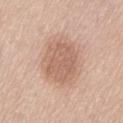{"biopsy_status": "not biopsied; imaged during a skin examination", "image": {"source": "total-body photography crop", "field_of_view_mm": 15}, "patient": {"sex": "female", "age_approx": 65}, "automated_metrics": {"area_mm2_approx": 17.0, "eccentricity": 0.65, "shape_asymmetry": 0.15, "color_variation_0_10": 2.5, "peripheral_color_asymmetry": 1.0, "nevus_likeness_0_100": 20, "lesion_detection_confidence_0_100": 100}, "site": "abdomen"}